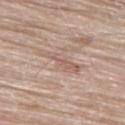biopsy status — imaged on a skin check; not biopsied
image — total-body-photography crop, ~15 mm field of view
subject — male, aged around 80
anatomic site — the left thigh
automated metrics — an automated nevus-likeness rating near 0 out of 100 and lesion-presence confidence of about 50/100
lighting — white-light